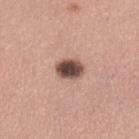{
  "biopsy_status": "not biopsied; imaged during a skin examination",
  "lesion_size": {
    "long_diameter_mm_approx": 3.0
  },
  "patient": {
    "sex": "female",
    "age_approx": 30
  },
  "site": "left lower leg",
  "image": {
    "source": "total-body photography crop",
    "field_of_view_mm": 15
  },
  "lighting": "white-light"
}